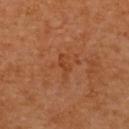Q: Was this lesion biopsied?
A: imaged on a skin check; not biopsied
Q: What is the imaging modality?
A: 15 mm crop, total-body photography
Q: What is the anatomic site?
A: the upper back
Q: Who is the patient?
A: female, roughly 65 years of age